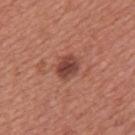Impression: This lesion was catalogued during total-body skin photography and was not selected for biopsy. Clinical summary: The lesion is located on the chest. Approximately 3 mm at its widest. Cropped from a whole-body photographic skin survey; the tile spans about 15 mm. The subject is a male aged approximately 45.